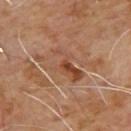| field | value |
|---|---|
| follow-up | total-body-photography surveillance lesion; no biopsy |
| location | the upper back |
| acquisition | 15 mm crop, total-body photography |
| subject | male, about 65 years old |